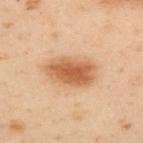{
  "biopsy_status": "not biopsied; imaged during a skin examination",
  "lesion_size": {
    "long_diameter_mm_approx": 5.5
  },
  "lighting": "cross-polarized",
  "patient": {
    "sex": "male",
    "age_approx": 50
  },
  "automated_metrics": {
    "cielab_L": 49,
    "cielab_a": 20,
    "cielab_b": 32,
    "vs_skin_darker_L": 11.0,
    "vs_skin_contrast_norm": 9.0,
    "lesion_detection_confidence_0_100": 100
  },
  "site": "upper back",
  "image": {
    "source": "total-body photography crop",
    "field_of_view_mm": 15
  }
}